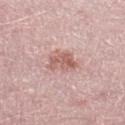Part of a total-body skin-imaging series; this lesion was reviewed on a skin check and was not flagged for biopsy.
From the left thigh.
Cropped from a total-body skin-imaging series; the visible field is about 15 mm.
The subject is a male approximately 50 years of age.